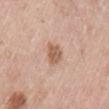  biopsy_status: not biopsied; imaged during a skin examination